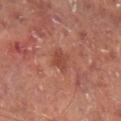The lesion was tiled from a total-body skin photograph and was not biopsied.
Cropped from a total-body skin-imaging series; the visible field is about 15 mm.
An algorithmic analysis of the crop reported a lesion area of about 4.5 mm². And it measured internal color variation of about 2 on a 0–10 scale and peripheral color asymmetry of about 1. The software also gave a nevus-likeness score of about 10/100 and a lesion-detection confidence of about 100/100.
On the right lower leg.
A male subject roughly 65 years of age.
Imaged with cross-polarized lighting.